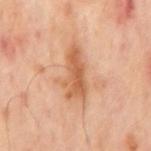<case>
  <biopsy_status>not biopsied; imaged during a skin examination</biopsy_status>
  <automated_metrics>
    <area_mm2_approx>12.0</area_mm2_approx>
    <eccentricity>0.9</eccentricity>
    <shape_asymmetry>0.35</shape_asymmetry>
  </automated_metrics>
  <image>
    <source>total-body photography crop</source>
    <field_of_view_mm>15</field_of_view_mm>
  </image>
  <lesion_size>
    <long_diameter_mm_approx>6.5</long_diameter_mm_approx>
  </lesion_size>
  <patient>
    <sex>male</sex>
    <age_approx>65</age_approx>
  </patient>
  <site>mid back</site>
</case>